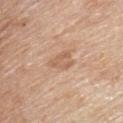{
  "biopsy_status": "not biopsied; imaged during a skin examination",
  "lighting": "white-light",
  "automated_metrics": {
    "area_mm2_approx": 4.5,
    "eccentricity": 0.55,
    "cielab_L": 60,
    "cielab_a": 20,
    "cielab_b": 32,
    "vs_skin_darker_L": 8.0,
    "vs_skin_contrast_norm": 5.5
  },
  "patient": {
    "sex": "male",
    "age_approx": 55
  },
  "lesion_size": {
    "long_diameter_mm_approx": 3.0
  },
  "site": "chest",
  "image": {
    "source": "total-body photography crop",
    "field_of_view_mm": 15
  }
}